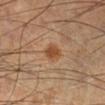Recorded during total-body skin imaging; not selected for excision or biopsy.
The tile uses cross-polarized illumination.
This image is a 15 mm lesion crop taken from a total-body photograph.
Automated image analysis of the tile measured an outline eccentricity of about 0.7 (0 = round, 1 = elongated) and two-axis asymmetry of about 0.2. And it measured a mean CIELAB color near L≈48 a*≈22 b*≈35, a lesion–skin lightness drop of about 9, and a normalized border contrast of about 8. And it measured an automated nevus-likeness rating near 95 out of 100 and a detector confidence of about 100 out of 100 that the crop contains a lesion.
The lesion is located on the right lower leg.
Longest diameter approximately 2.5 mm.
A male subject, aged 53 to 57.